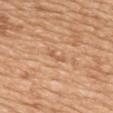Notes:
– workup · catalogued during a skin exam; not biopsied